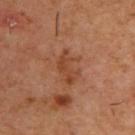Clinical impression:
This lesion was catalogued during total-body skin photography and was not selected for biopsy.
Acquisition and patient details:
Located on the upper back. This is a cross-polarized tile. Approximately 4.5 mm at its widest. Cropped from a total-body skin-imaging series; the visible field is about 15 mm. A male subject, about 55 years old.